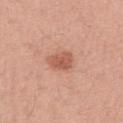Case summary:
- follow-up · no biopsy performed (imaged during a skin exam)
- subject · female, aged approximately 35
- lesion size · ≈3 mm
- location · the right upper arm
- tile lighting · white-light illumination
- image · ~15 mm tile from a whole-body skin photo